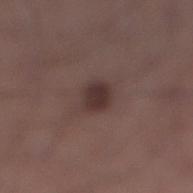A male subject, aged around 55.
A 15 mm close-up tile from a total-body photography series done for melanoma screening.
Located on the right lower leg.